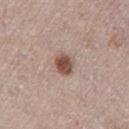Notes:
– workup · no biopsy performed (imaged during a skin exam)
– image-analysis metrics · a lesion color around L≈51 a*≈19 b*≈25 in CIELAB and a normalized border contrast of about 10; a border-irregularity rating of about 1.5/10, internal color variation of about 3.5 on a 0–10 scale, and peripheral color asymmetry of about 1; a nevus-likeness score of about 100/100 and a lesion-detection confidence of about 100/100
– tile lighting · white-light
– size · ≈3 mm
– image source · ~15 mm tile from a whole-body skin photo
– patient · male, approximately 75 years of age
– anatomic site · the leg